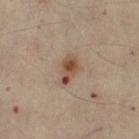{
  "biopsy_status": "not biopsied; imaged during a skin examination",
  "automated_metrics": {
    "area_mm2_approx": 5.5,
    "eccentricity": 0.85,
    "shape_asymmetry": 0.25,
    "cielab_L": 48,
    "cielab_a": 18,
    "cielab_b": 29,
    "vs_skin_darker_L": 11.0,
    "vs_skin_contrast_norm": 9.0,
    "nevus_likeness_0_100": 10,
    "lesion_detection_confidence_0_100": 100
  },
  "lesion_size": {
    "long_diameter_mm_approx": 3.5
  },
  "image": {
    "source": "total-body photography crop",
    "field_of_view_mm": 15
  },
  "patient": {
    "sex": "female",
    "age_approx": 60
  },
  "site": "right thigh"
}